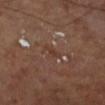Q: Was a biopsy performed?
A: catalogued during a skin exam; not biopsied
Q: How was this image acquired?
A: ~15 mm crop, total-body skin-cancer survey
Q: Lesion location?
A: the left lower leg
Q: What did automated image analysis measure?
A: an eccentricity of roughly 0.9 and a symmetry-axis asymmetry near 0.45; a border-irregularity index near 4.5/10 and a within-lesion color-variation index near 0/10; a detector confidence of about 65 out of 100 that the crop contains a lesion
Q: How was the tile lit?
A: cross-polarized illumination
Q: What is the lesion's diameter?
A: ≈2.5 mm
Q: Who is the patient?
A: male, aged 68–72